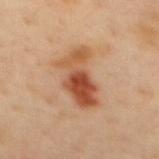| key | value |
|---|---|
| follow-up | catalogued during a skin exam; not biopsied |
| body site | the mid back |
| subject | male, about 40 years old |
| image | total-body-photography crop, ~15 mm field of view |
| lesion diameter | ≈6 mm |
| lighting | cross-polarized |